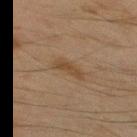  biopsy_status: not biopsied; imaged during a skin examination
  lighting: cross-polarized
  patient:
    sex: male
    age_approx: 45
  image:
    source: total-body photography crop
    field_of_view_mm: 15
  automated_metrics:
    color_variation_0_10: 1.5
    peripheral_color_asymmetry: 0.5
    nevus_likeness_0_100: 50
    lesion_detection_confidence_0_100: 100
  site: back
  lesion_size:
    long_diameter_mm_approx: 3.5